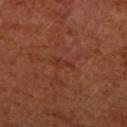Imaged with cross-polarized lighting.
Approximately 2.5 mm at its widest.
The lesion is on the left forearm.
A female subject roughly 65 years of age.
A region of skin cropped from a whole-body photographic capture, roughly 15 mm wide.
An algorithmic analysis of the crop reported an area of roughly 2 mm², an outline eccentricity of about 0.95 (0 = round, 1 = elongated), and a symmetry-axis asymmetry near 0.55.